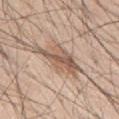Findings:
• subject — male, aged 43–47
• anatomic site — the mid back
• image source — ~15 mm tile from a whole-body skin photo
• illumination — white-light